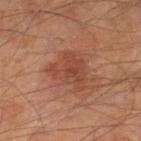Impression: The lesion was photographed on a routine skin check and not biopsied; there is no pathology result. Context: A region of skin cropped from a whole-body photographic capture, roughly 15 mm wide. Captured under cross-polarized illumination. The lesion is on the right lower leg. A male patient, in their mid- to late 60s. The lesion's longest dimension is about 5 mm.